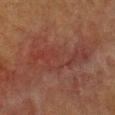{
  "biopsy_status": "not biopsied; imaged during a skin examination",
  "patient": {
    "sex": "female",
    "age_approx": 40
  },
  "lesion_size": {
    "long_diameter_mm_approx": 8.5
  },
  "lighting": "cross-polarized",
  "automated_metrics": {
    "area_mm2_approx": 23.0,
    "eccentricity": 0.95,
    "shape_asymmetry": 0.4,
    "cielab_L": 31,
    "cielab_a": 21,
    "cielab_b": 22,
    "vs_skin_darker_L": 4.0,
    "vs_skin_contrast_norm": 5.0,
    "border_irregularity_0_10": 6.0,
    "color_variation_0_10": 4.5,
    "peripheral_color_asymmetry": 1.5
  },
  "image": {
    "source": "total-body photography crop",
    "field_of_view_mm": 15
  },
  "site": "chest"
}